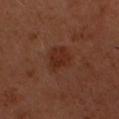| field | value |
|---|---|
| workup | imaged on a skin check; not biopsied |
| size | ~4 mm (longest diameter) |
| lighting | cross-polarized illumination |
| image | ~15 mm tile from a whole-body skin photo |
| image-analysis metrics | a mean CIELAB color near L≈29 a*≈23 b*≈27, roughly 6 lightness units darker than nearby skin, and a lesion-to-skin contrast of about 6.5 (normalized; higher = more distinct); an automated nevus-likeness rating near 25 out of 100 and a lesion-detection confidence of about 100/100 |
| body site | the head or neck |
| patient | male, aged 48 to 52 |